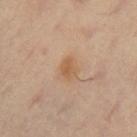| feature | finding |
|---|---|
| notes | catalogued during a skin exam; not biopsied |
| subject | female, about 45 years old |
| image | ~15 mm crop, total-body skin-cancer survey |
| illumination | cross-polarized illumination |
| site | the right thigh |